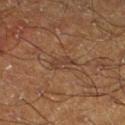Clinical impression: No biopsy was performed on this lesion — it was imaged during a full skin examination and was not determined to be concerning. Image and clinical context: This is a cross-polarized tile. Cropped from a whole-body photographic skin survey; the tile spans about 15 mm. The lesion is located on the leg. Measured at roughly 3 mm in maximum diameter. Automated image analysis of the tile measured a lesion area of about 2.5 mm², an outline eccentricity of about 0.95 (0 = round, 1 = elongated), and a symmetry-axis asymmetry near 0.4. The analysis additionally found a border-irregularity index near 4.5/10 and a peripheral color-asymmetry measure near 0. The software also gave an automated nevus-likeness rating near 0 out of 100 and a detector confidence of about 55 out of 100 that the crop contains a lesion. A male patient aged around 65.